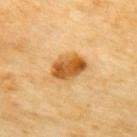Assessment: Part of a total-body skin-imaging series; this lesion was reviewed on a skin check and was not flagged for biopsy. Clinical summary: A female subject aged 68–72. The recorded lesion diameter is about 4.5 mm. Cropped from a total-body skin-imaging series; the visible field is about 15 mm. Located on the upper back.